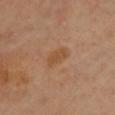| feature | finding |
|---|---|
| notes | imaged on a skin check; not biopsied |
| patient | female, aged 38–42 |
| acquisition | total-body-photography crop, ~15 mm field of view |
| body site | the chest |